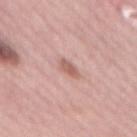Findings:
* follow-up: total-body-photography surveillance lesion; no biopsy
* lighting: white-light
* patient: female, aged 63–67
* location: the mid back
* image-analysis metrics: an area of roughly 3 mm², an outline eccentricity of about 0.9 (0 = round, 1 = elongated), and two-axis asymmetry of about 0.35
* imaging modality: 15 mm crop, total-body photography
* lesion diameter: about 3 mm